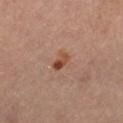  biopsy_status: not biopsied; imaged during a skin examination
  patient:
    sex: female
    age_approx: 45
  site: left thigh
  automated_metrics:
    nevus_likeness_0_100: 90
    lesion_detection_confidence_0_100: 100
  lighting: cross-polarized
  lesion_size:
    long_diameter_mm_approx: 2.5
  image:
    source: total-body photography crop
    field_of_view_mm: 15